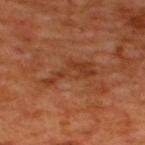{"biopsy_status": "not biopsied; imaged during a skin examination", "lesion_size": {"long_diameter_mm_approx": 6.0}, "lighting": "cross-polarized", "image": {"source": "total-body photography crop", "field_of_view_mm": 15}, "automated_metrics": {"cielab_L": 39, "cielab_a": 27, "cielab_b": 35, "vs_skin_darker_L": 7.0, "vs_skin_contrast_norm": 6.5, "nevus_likeness_0_100": 0}, "site": "back", "patient": {"sex": "male", "age_approx": 50}}